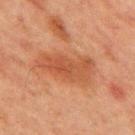A male patient approximately 65 years of age. Approximately 6.5 mm at its widest. A 15 mm close-up tile from a total-body photography series done for melanoma screening. Located on the front of the torso. Automated tile analysis of the lesion measured a border-irregularity rating of about 4/10, a color-variation rating of about 3.5/10, and peripheral color asymmetry of about 1.5. The tile uses cross-polarized illumination.